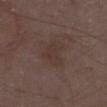Clinical summary:
Located on the right lower leg. This is a white-light tile. Automated image analysis of the tile measured a lesion color around L≈34 a*≈15 b*≈20 in CIELAB and a normalized lesion–skin contrast near 4.5. It also reported a color-variation rating of about 2/10 and peripheral color asymmetry of about 0.5. A male subject, about 70 years old. A 15 mm close-up extracted from a 3D total-body photography capture. About 3.5 mm across.